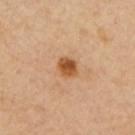biopsy status = imaged on a skin check; not biopsied | site = the upper back | lesion diameter = ≈2.5 mm | automated metrics = a lesion area of about 4.5 mm², an eccentricity of roughly 0.55, and two-axis asymmetry of about 0.15; an average lesion color of about L≈54 a*≈24 b*≈40 (CIELAB) | imaging modality = ~15 mm crop, total-body skin-cancer survey | patient = female, in their mid- to late 60s | lighting = cross-polarized.